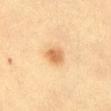Q: Was a biopsy performed?
A: imaged on a skin check; not biopsied
Q: What is the anatomic site?
A: the abdomen
Q: What is the imaging modality?
A: 15 mm crop, total-body photography
Q: What lighting was used for the tile?
A: cross-polarized
Q: How large is the lesion?
A: ≈2.5 mm
Q: Who is the patient?
A: female, approximately 55 years of age